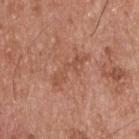Q: Was a biopsy performed?
A: catalogued during a skin exam; not biopsied
Q: Automated lesion metrics?
A: a mean CIELAB color near L≈52 a*≈24 b*≈31, a lesion–skin lightness drop of about 6, and a normalized border contrast of about 5
Q: What lighting was used for the tile?
A: white-light
Q: What kind of image is this?
A: ~15 mm crop, total-body skin-cancer survey
Q: What is the anatomic site?
A: the upper back
Q: Patient demographics?
A: male, in their mid- to late 50s
Q: Lesion size?
A: ≈5 mm